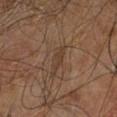workup: catalogued during a skin exam; not biopsied | automated metrics: an outline eccentricity of about 0.95 (0 = round, 1 = elongated); roughly 6 lightness units darker than nearby skin; border irregularity of about 5.5 on a 0–10 scale, internal color variation of about 0 on a 0–10 scale, and peripheral color asymmetry of about 0; a classifier nevus-likeness of about 0/100 and a lesion-detection confidence of about 50/100 | tile lighting: cross-polarized | patient: male, aged approximately 60 | lesion diameter: about 3 mm | body site: the right leg | acquisition: ~15 mm tile from a whole-body skin photo.